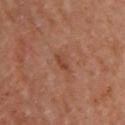  biopsy_status: not biopsied; imaged during a skin examination
  site: upper back
  lesion_size:
    long_diameter_mm_approx: 3.0
  image:
    source: total-body photography crop
    field_of_view_mm: 15
  automated_metrics:
    cielab_L: 46
    cielab_a: 24
    cielab_b: 33
    vs_skin_contrast_norm: 6.0
    border_irregularity_0_10: 2.5
    color_variation_0_10: 3.0
    peripheral_color_asymmetry: 1.0
  patient:
    sex: female
    age_approx: 60
  lighting: cross-polarized